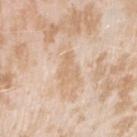Findings:
• notes · total-body-photography surveillance lesion; no biopsy
• illumination · white-light
• site · the right upper arm
• image-analysis metrics · an outline eccentricity of about 0.85 (0 = round, 1 = elongated) and a shape-asymmetry score of about 0.45 (0 = symmetric); a lesion-detection confidence of about 90/100
• image source · total-body-photography crop, ~15 mm field of view
• subject · female, approximately 25 years of age